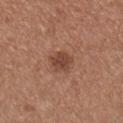From the right lower leg.
The recorded lesion diameter is about 3 mm.
A region of skin cropped from a whole-body photographic capture, roughly 15 mm wide.
The total-body-photography lesion software estimated a border-irregularity index near 3/10 and radial color variation of about 0.5. The software also gave a nevus-likeness score of about 85/100.
A female subject aged approximately 55.